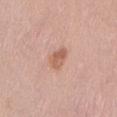Findings:
- workup · imaged on a skin check; not biopsied
- site · the mid back
- subject · female, approximately 40 years of age
- image · ~15 mm tile from a whole-body skin photo